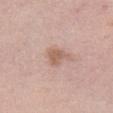Clinical impression: The lesion was photographed on a routine skin check and not biopsied; there is no pathology result. Context: This image is a 15 mm lesion crop taken from a total-body photograph. The lesion is on the left thigh. The patient is a male aged 38 to 42.